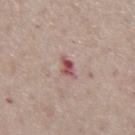The lesion was tiled from a total-body skin photograph and was not biopsied. About 3 mm across. Imaged with white-light lighting. The total-body-photography lesion software estimated a footprint of about 3 mm², an eccentricity of roughly 0.85, and a shape-asymmetry score of about 0.35 (0 = symmetric). And it measured a border-irregularity index near 3.5/10 and peripheral color asymmetry of about 1.5. The analysis additionally found a classifier nevus-likeness of about 0/100 and lesion-presence confidence of about 100/100. The lesion is on the front of the torso. The subject is a male about 75 years old. A region of skin cropped from a whole-body photographic capture, roughly 15 mm wide.